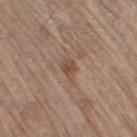Findings:
– image-analysis metrics — a normalized border contrast of about 7; border irregularity of about 3.5 on a 0–10 scale, internal color variation of about 2.5 on a 0–10 scale, and peripheral color asymmetry of about 1
– body site — the right thigh
– tile lighting — white-light illumination
– acquisition — 15 mm crop, total-body photography
– patient — male, aged 68–72